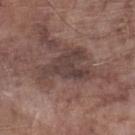A close-up tile cropped from a whole-body skin photograph, about 15 mm across. The subject is a male in their mid-70s. The lesion is located on the leg.